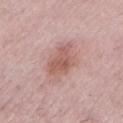Findings:
* workup · no biopsy performed (imaged during a skin exam)
* size · ≈4.5 mm
* site · the left lower leg
* image · ~15 mm tile from a whole-body skin photo
* patient · female, in their mid-60s
* lighting · white-light